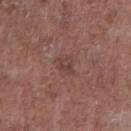Case summary:
– workup — imaged on a skin check; not biopsied
– subject — male, approximately 70 years of age
– lesion diameter — about 2.5 mm
– automated metrics — an area of roughly 3.5 mm², a shape eccentricity near 0.7, and a symmetry-axis asymmetry near 0.2; a lesion color around L≈41 a*≈19 b*≈22 in CIELAB, roughly 6 lightness units darker than nearby skin, and a normalized lesion–skin contrast near 5.5; internal color variation of about 3 on a 0–10 scale; a nevus-likeness score of about 0/100
– image source — total-body-photography crop, ~15 mm field of view
– illumination — white-light
– body site — the front of the torso